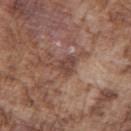Assessment: The lesion was photographed on a routine skin check and not biopsied; there is no pathology result. Acquisition and patient details: About 2.5 mm across. The tile uses white-light illumination. From the right upper arm. This image is a 15 mm lesion crop taken from a total-body photograph. The patient is a male aged approximately 75.